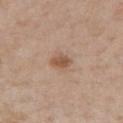automated metrics = an eccentricity of roughly 0.7 and a symmetry-axis asymmetry near 0.25; radial color variation of about 0.5 | patient = female, in their mid-40s | lesion diameter = ≈2.5 mm | anatomic site = the chest | acquisition = 15 mm crop, total-body photography.